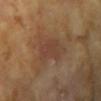The lesion was photographed on a routine skin check and not biopsied; there is no pathology result. A female patient aged 73–77. The tile uses cross-polarized illumination. A 15 mm close-up tile from a total-body photography series done for melanoma screening. Approximately 4 mm at its widest. On the right upper arm.